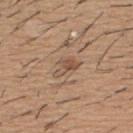No biopsy was performed on this lesion — it was imaged during a full skin examination and was not determined to be concerning. Located on the upper back. The subject is a male aged 58–62. A region of skin cropped from a whole-body photographic capture, roughly 15 mm wide.